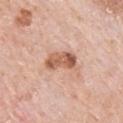notes = no biopsy performed (imaged during a skin exam); lesion size = about 4 mm; patient = male, aged 78 to 82; body site = the back; tile lighting = white-light illumination; image-analysis metrics = a classifier nevus-likeness of about 60/100; imaging modality = 15 mm crop, total-body photography.